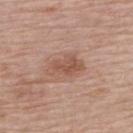Recorded during total-body skin imaging; not selected for excision or biopsy. Longest diameter approximately 4.5 mm. Imaged with white-light lighting. On the upper back. The patient is a male about 85 years old or older. A region of skin cropped from a whole-body photographic capture, roughly 15 mm wide.